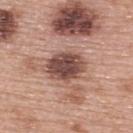acquisition = 15 mm crop, total-body photography
body site = the back
subject = female, aged 58 to 62